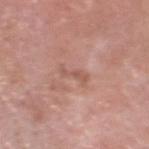workup: imaged on a skin check; not biopsied | TBP lesion metrics: a lesion area of about 3 mm² and a shape eccentricity near 0.95; an average lesion color of about L≈56 a*≈23 b*≈27 (CIELAB) and roughly 7 lightness units darker than nearby skin; an automated nevus-likeness rating near 0 out of 100 and a lesion-detection confidence of about 95/100 | body site: the head or neck | lesion diameter: ~3.5 mm (longest diameter) | patient: male, aged 58–62 | illumination: white-light | image source: 15 mm crop, total-body photography.